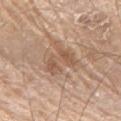Assessment:
The lesion was tiled from a total-body skin photograph and was not biopsied.
Context:
The total-body-photography lesion software estimated an area of roughly 7.5 mm² and a shape-asymmetry score of about 0.5 (0 = symmetric). The analysis additionally found a border-irregularity rating of about 7/10, a color-variation rating of about 2.5/10, and a peripheral color-asymmetry measure near 1. A 15 mm crop from a total-body photograph taken for skin-cancer surveillance. The lesion is on the right upper arm. The tile uses white-light illumination. A male patient, approximately 80 years of age.